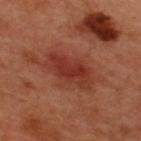No biopsy was performed on this lesion — it was imaged during a full skin examination and was not determined to be concerning. The lesion is located on the upper back. A 15 mm close-up tile from a total-body photography series done for melanoma screening. The tile uses cross-polarized illumination. The patient is a male aged 48 to 52.